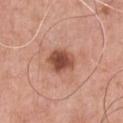notes = imaged on a skin check; not biopsied
automated lesion analysis = roughly 15 lightness units darker than nearby skin; an automated nevus-likeness rating near 90 out of 100 and a detector confidence of about 100 out of 100 that the crop contains a lesion
patient = male, about 60 years old
image = total-body-photography crop, ~15 mm field of view
diameter = ≈3.5 mm
lighting = white-light illumination
location = the chest